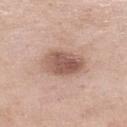- follow-up: no biopsy performed (imaged during a skin exam)
- location: the left lower leg
- patient: female, aged around 55
- image: 15 mm crop, total-body photography